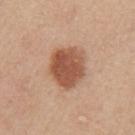| field | value |
|---|---|
| location | the arm |
| image | ~15 mm tile from a whole-body skin photo |
| diameter | ~5 mm (longest diameter) |
| illumination | white-light |
| subject | female, aged around 45 |
| image-analysis metrics | a footprint of about 16 mm², an outline eccentricity of about 0.5 (0 = round, 1 = elongated), and a symmetry-axis asymmetry near 0.15; a border-irregularity rating of about 1.5/10, a color-variation rating of about 4/10, and peripheral color asymmetry of about 1.5 |